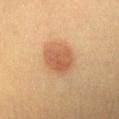Recorded during total-body skin imaging; not selected for excision or biopsy.
A close-up tile cropped from a whole-body skin photograph, about 15 mm across.
Imaged with cross-polarized lighting.
The lesion is on the chest.
A female subject aged 38–42.